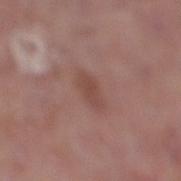This lesion was catalogued during total-body skin photography and was not selected for biopsy. This is a white-light tile. Cropped from a total-body skin-imaging series; the visible field is about 15 mm. A female patient, aged 83 or older. The lesion is on the right lower leg. Measured at roughly 3 mm in maximum diameter.